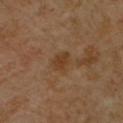Q: Was a biopsy performed?
A: imaged on a skin check; not biopsied
Q: Where on the body is the lesion?
A: the right upper arm
Q: What kind of image is this?
A: 15 mm crop, total-body photography
Q: What are the patient's age and sex?
A: female, in their mid- to late 50s
Q: Illumination type?
A: cross-polarized